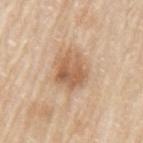<case>
<biopsy_status>not biopsied; imaged during a skin examination</biopsy_status>
<site>left upper arm</site>
<patient>
  <sex>male</sex>
  <age_approx>80</age_approx>
</patient>
<image>
  <source>total-body photography crop</source>
  <field_of_view_mm>15</field_of_view_mm>
</image>
<lesion_size>
  <long_diameter_mm_approx>4.5</long_diameter_mm_approx>
</lesion_size>
</case>